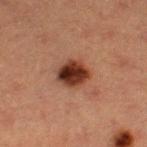notes: imaged on a skin check; not biopsied
patient: female, roughly 40 years of age
tile lighting: cross-polarized illumination
site: the left thigh
diameter: ~4 mm (longest diameter)
imaging modality: total-body-photography crop, ~15 mm field of view